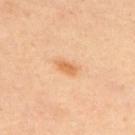Recorded during total-body skin imaging; not selected for excision or biopsy. Captured under cross-polarized illumination. The lesion is on the upper back. Cropped from a total-body skin-imaging series; the visible field is about 15 mm. Automated image analysis of the tile measured a footprint of about 3.5 mm² and an eccentricity of roughly 0.85. The subject is a male roughly 45 years of age. Measured at roughly 3 mm in maximum diameter.